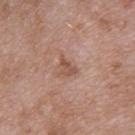Captured during whole-body skin photography for melanoma surveillance; the lesion was not biopsied. A region of skin cropped from a whole-body photographic capture, roughly 15 mm wide. Approximately 2.5 mm at its widest. A male subject, approximately 50 years of age. From the upper back. Imaged with white-light lighting. Automated image analysis of the tile measured an area of roughly 3.5 mm² and a symmetry-axis asymmetry near 0.45. And it measured a normalized lesion–skin contrast near 6.5. The software also gave border irregularity of about 4 on a 0–10 scale and a within-lesion color-variation index near 2/10. And it measured a detector confidence of about 100 out of 100 that the crop contains a lesion.